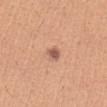* illumination — white-light
* body site — the abdomen
* subject — female, aged approximately 40
* lesion size — about 2 mm
* acquisition — total-body-photography crop, ~15 mm field of view